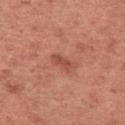The lesion was photographed on a routine skin check and not biopsied; there is no pathology result. From the arm. The total-body-photography lesion software estimated a lesion area of about 3 mm² and an eccentricity of roughly 0.85. It also reported an average lesion color of about L≈50 a*≈28 b*≈29 (CIELAB), roughly 9 lightness units darker than nearby skin, and a normalized lesion–skin contrast near 6. It also reported a border-irregularity index near 2.5/10. And it measured a nevus-likeness score of about 15/100 and lesion-presence confidence of about 100/100. Cropped from a whole-body photographic skin survey; the tile spans about 15 mm. A female subject approximately 50 years of age. Captured under white-light illumination. Approximately 3 mm at its widest.